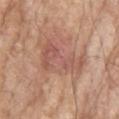The lesion was tiled from a total-body skin photograph and was not biopsied.
About 6 mm across.
Imaged with white-light lighting.
On the back.
An algorithmic analysis of the crop reported border irregularity of about 6.5 on a 0–10 scale and a peripheral color-asymmetry measure near 1.5. And it measured a nevus-likeness score of about 5/100 and a lesion-detection confidence of about 100/100.
The patient is a male approximately 85 years of age or older.
A 15 mm close-up tile from a total-body photography series done for melanoma screening.